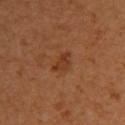Captured during whole-body skin photography for melanoma surveillance; the lesion was not biopsied. Located on the upper back. Automated image analysis of the tile measured a lesion area of about 4 mm² and an eccentricity of roughly 0.8. It also reported a lesion color around L≈36 a*≈24 b*≈33 in CIELAB, roughly 7 lightness units darker than nearby skin, and a normalized border contrast of about 6.5. It also reported a border-irregularity rating of about 3.5/10, a color-variation rating of about 2.5/10, and radial color variation of about 1. The software also gave an automated nevus-likeness rating near 10 out of 100 and a detector confidence of about 100 out of 100 that the crop contains a lesion. Captured under cross-polarized illumination. A lesion tile, about 15 mm wide, cut from a 3D total-body photograph. The patient is a female aged approximately 35.